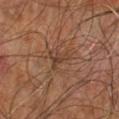| field | value |
|---|---|
| notes | imaged on a skin check; not biopsied |
| automated metrics | a lesion-detection confidence of about 60/100 |
| site | the right leg |
| diameter | about 3 mm |
| patient | male, aged 58–62 |
| image source | ~15 mm tile from a whole-body skin photo |